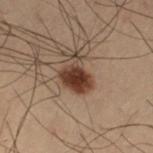biopsy status — total-body-photography surveillance lesion; no biopsy
image — ~15 mm crop, total-body skin-cancer survey
illumination — cross-polarized illumination
TBP lesion metrics — an area of roughly 9 mm², an outline eccentricity of about 0.6 (0 = round, 1 = elongated), and a shape-asymmetry score of about 0.4 (0 = symmetric); an average lesion color of about L≈30 a*≈15 b*≈22 (CIELAB) and a normalized border contrast of about 11.5; a classifier nevus-likeness of about 100/100 and a detector confidence of about 100 out of 100 that the crop contains a lesion
lesion size — ~4 mm (longest diameter)
location — the left thigh
patient — male, about 55 years old